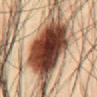Clinical impression: No biopsy was performed on this lesion — it was imaged during a full skin examination and was not determined to be concerning. Image and clinical context: The tile uses cross-polarized illumination. Cropped from a whole-body photographic skin survey; the tile spans about 15 mm. On the front of the torso. The subject is a male in their mid-30s.